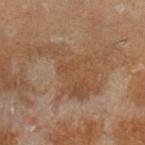The lesion was photographed on a routine skin check and not biopsied; there is no pathology result.
Longest diameter approximately 9 mm.
A lesion tile, about 15 mm wide, cut from a 3D total-body photograph.
A male patient, approximately 30 years of age.
Captured under cross-polarized illumination.
The lesion is on the leg.
Automated image analysis of the tile measured an area of roughly 21 mm², a shape eccentricity near 0.9, and two-axis asymmetry of about 0.6. And it measured an average lesion color of about L≈38 a*≈15 b*≈26 (CIELAB), roughly 5 lightness units darker than nearby skin, and a normalized lesion–skin contrast near 5.5. And it measured a border-irregularity rating of about 9.5/10, a color-variation rating of about 3/10, and a peripheral color-asymmetry measure near 1. And it measured an automated nevus-likeness rating near 0 out of 100 and lesion-presence confidence of about 90/100.